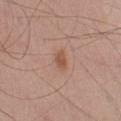| feature | finding |
|---|---|
| image | ~15 mm crop, total-body skin-cancer survey |
| body site | the arm |
| subject | male, about 65 years old |